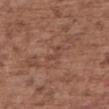notes: catalogued during a skin exam; not biopsied
imaging modality: ~15 mm crop, total-body skin-cancer survey
lesion diameter: about 2.5 mm
automated lesion analysis: a lesion area of about 2.5 mm² and a symmetry-axis asymmetry near 0.6
subject: female, roughly 75 years of age
illumination: white-light illumination
body site: the upper back